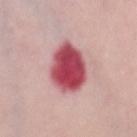Impression: Imaged during a routine full-body skin examination; the lesion was not biopsied and no histopathology is available. Context: Captured under white-light illumination. A female patient, roughly 65 years of age. The lesion is on the chest. A roughly 15 mm field-of-view crop from a total-body skin photograph. The recorded lesion diameter is about 5.5 mm.